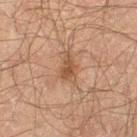Imaged during a routine full-body skin examination; the lesion was not biopsied and no histopathology is available. A male subject about 60 years old. From the left thigh. The total-body-photography lesion software estimated an area of roughly 4.5 mm² and a shape eccentricity near 0.85. The software also gave a mean CIELAB color near L≈39 a*≈16 b*≈26, about 8 CIELAB-L* units darker than the surrounding skin, and a lesion-to-skin contrast of about 7 (normalized; higher = more distinct). Imaged with cross-polarized lighting. About 3.5 mm across. A region of skin cropped from a whole-body photographic capture, roughly 15 mm wide.